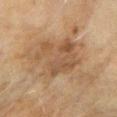Context: A female patient, aged around 60. From the arm. A close-up tile cropped from a whole-body skin photograph, about 15 mm across.